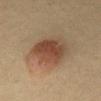Case summary:
– follow-up — catalogued during a skin exam; not biopsied